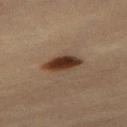A 15 mm crop from a total-body photograph taken for skin-cancer surveillance. On the right thigh. An algorithmic analysis of the crop reported an area of roughly 7 mm², a shape eccentricity near 0.9, and a symmetry-axis asymmetry near 0.25. The software also gave a border-irregularity rating of about 2.5/10, a color-variation rating of about 3.5/10, and a peripheral color-asymmetry measure near 1. The software also gave lesion-presence confidence of about 100/100. A female patient, in their mid- to late 40s.